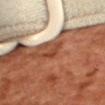The subject is a female approximately 55 years of age. A close-up tile cropped from a whole-body skin photograph, about 15 mm across. Located on the back. The tile uses cross-polarized illumination. Longest diameter approximately 2.5 mm.